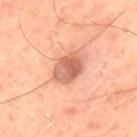Clinical impression:
Imaged during a routine full-body skin examination; the lesion was not biopsied and no histopathology is available.
Acquisition and patient details:
On the upper back. The subject is a male aged approximately 50. An algorithmic analysis of the crop reported a mean CIELAB color near L≈63 a*≈27 b*≈34, a lesion–skin lightness drop of about 13, and a normalized border contrast of about 8. The analysis additionally found a classifier nevus-likeness of about 60/100. A region of skin cropped from a whole-body photographic capture, roughly 15 mm wide. Measured at roughly 4 mm in maximum diameter. Captured under cross-polarized illumination.